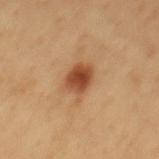Impression: This lesion was catalogued during total-body skin photography and was not selected for biopsy. Image and clinical context: Captured under cross-polarized illumination. A region of skin cropped from a whole-body photographic capture, roughly 15 mm wide. Located on the mid back. About 4 mm across. A male patient roughly 50 years of age. An algorithmic analysis of the crop reported a lesion color around L≈49 a*≈24 b*≈36 in CIELAB, about 13 CIELAB-L* units darker than the surrounding skin, and a normalized border contrast of about 9.5. And it measured a classifier nevus-likeness of about 100/100 and a lesion-detection confidence of about 100/100.